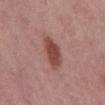Part of a total-body skin-imaging series; this lesion was reviewed on a skin check and was not flagged for biopsy.
Longest diameter approximately 4 mm.
The lesion is located on the mid back.
A male patient aged 58 to 62.
A close-up tile cropped from a whole-body skin photograph, about 15 mm across.
This is a white-light tile.